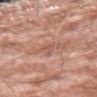The lesion was tiled from a total-body skin photograph and was not biopsied.
A male subject roughly 60 years of age.
On the arm.
The lesion's longest dimension is about 2.5 mm.
This image is a 15 mm lesion crop taken from a total-body photograph.
An algorithmic analysis of the crop reported an eccentricity of roughly 0.85 and two-axis asymmetry of about 0.45. And it measured a lesion color around L≈56 a*≈21 b*≈27 in CIELAB and a lesion–skin lightness drop of about 7. The analysis additionally found a border-irregularity rating of about 4.5/10 and a color-variation rating of about 1.5/10.
This is a white-light tile.